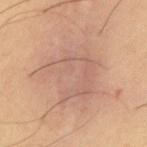Assessment:
Recorded during total-body skin imaging; not selected for excision or biopsy.
Acquisition and patient details:
From the abdomen. The subject is a male in their mid- to late 50s. A 15 mm close-up extracted from a 3D total-body photography capture. The recorded lesion diameter is about 8 mm. Imaged with cross-polarized lighting. Automated image analysis of the tile measured a footprint of about 35 mm² and an eccentricity of roughly 0.5.